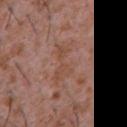follow-up: imaged on a skin check; not biopsied | subject: male, aged 43–47 | location: the chest | lighting: white-light illumination | acquisition: 15 mm crop, total-body photography | size: ≈6 mm | automated metrics: a footprint of about 10 mm², a shape eccentricity near 0.9, and a symmetry-axis asymmetry near 0.55; a border-irregularity rating of about 8/10; an automated nevus-likeness rating near 0 out of 100 and a detector confidence of about 50 out of 100 that the crop contains a lesion.